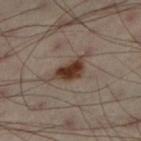| key | value |
|---|---|
| workup | total-body-photography surveillance lesion; no biopsy |
| body site | the left lower leg |
| subject | male, aged approximately 50 |
| acquisition | ~15 mm tile from a whole-body skin photo |